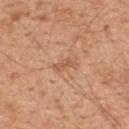Impression:
Part of a total-body skin-imaging series; this lesion was reviewed on a skin check and was not flagged for biopsy.
Background:
The patient is a male aged 43 to 47. Cropped from a total-body skin-imaging series; the visible field is about 15 mm. Located on the mid back. About 2.5 mm across. Automated tile analysis of the lesion measured an area of roughly 2.5 mm², an eccentricity of roughly 0.9, and a symmetry-axis asymmetry near 0.45. The analysis additionally found a lesion color around L≈57 a*≈22 b*≈35 in CIELAB and a lesion-to-skin contrast of about 5.5 (normalized; higher = more distinct). The software also gave a classifier nevus-likeness of about 0/100 and a lesion-detection confidence of about 100/100.